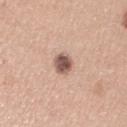<case>
  <image>
    <source>total-body photography crop</source>
    <field_of_view_mm>15</field_of_view_mm>
  </image>
  <site>left upper arm</site>
  <lighting>white-light</lighting>
  <automated_metrics>
    <area_mm2_approx>5.5</area_mm2_approx>
    <border_irregularity_0_10>1.0</border_irregularity_0_10>
    <peripheral_color_asymmetry>1.5</peripheral_color_asymmetry>
    <nevus_likeness_0_100>70</nevus_likeness_0_100>
    <lesion_detection_confidence_0_100>100</lesion_detection_confidence_0_100>
  </automated_metrics>
  <patient>
    <sex>female</sex>
    <age_approx>30</age_approx>
  </patient>
</case>